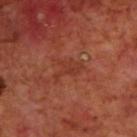- biopsy status: total-body-photography surveillance lesion; no biopsy
- patient: male, roughly 70 years of age
- anatomic site: the upper back
- imaging modality: total-body-photography crop, ~15 mm field of view
- lesion diameter: about 4 mm
- lighting: cross-polarized illumination